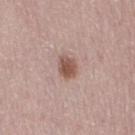No biopsy was performed on this lesion — it was imaged during a full skin examination and was not determined to be concerning.